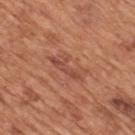Assessment:
No biopsy was performed on this lesion — it was imaged during a full skin examination and was not determined to be concerning.
Background:
Captured under white-light illumination. A male subject, aged approximately 65. Measured at roughly 4.5 mm in maximum diameter. On the mid back. A close-up tile cropped from a whole-body skin photograph, about 15 mm across.